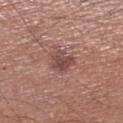biopsy status = no biopsy performed (imaged during a skin exam) | lesion diameter = about 3.5 mm | TBP lesion metrics = a color-variation rating of about 4/10 and a peripheral color-asymmetry measure near 1.5; a nevus-likeness score of about 0/100 and lesion-presence confidence of about 100/100 | location = the leg | imaging modality = 15 mm crop, total-body photography | illumination = white-light illumination | subject = male, aged 68 to 72.